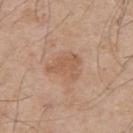  biopsy_status: not biopsied; imaged during a skin examination
  lesion_size:
    long_diameter_mm_approx: 4.0
  patient:
    sex: male
    age_approx: 55
  image:
    source: total-body photography crop
    field_of_view_mm: 15
  site: upper back
  lighting: white-light
  automated_metrics:
    shape_asymmetry: 0.4
    color_variation_0_10: 2.0
    peripheral_color_asymmetry: 1.0